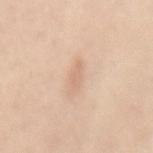The lesion was tiled from a total-body skin photograph and was not biopsied.
Captured under white-light illumination.
The subject is a female approximately 30 years of age.
A roughly 15 mm field-of-view crop from a total-body skin photograph.
Approximately 2.5 mm at its widest.
On the mid back.
An algorithmic analysis of the crop reported a footprint of about 2.5 mm² and an outline eccentricity of about 0.9 (0 = round, 1 = elongated). The software also gave an average lesion color of about L≈69 a*≈18 b*≈31 (CIELAB), a lesion–skin lightness drop of about 7, and a normalized border contrast of about 4.5.